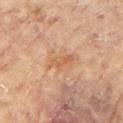Acquisition and patient details: The subject is a female roughly 80 years of age. On the arm. Longest diameter approximately 4 mm. Cropped from a whole-body photographic skin survey; the tile spans about 15 mm.